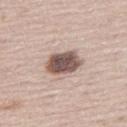notes — no biopsy performed (imaged during a skin exam); diameter — ≈4.5 mm; subject — male, aged 63–67; lighting — white-light illumination; image — ~15 mm crop, total-body skin-cancer survey; location — the leg.